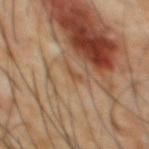Part of a total-body skin-imaging series; this lesion was reviewed on a skin check and was not flagged for biopsy. A 15 mm close-up extracted from a 3D total-body photography capture. The lesion's longest dimension is about 2 mm. On the chest. A male patient about 65 years old. The tile uses cross-polarized illumination.